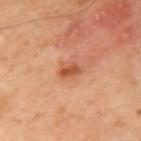biopsy_status: not biopsied; imaged during a skin examination
automated_metrics:
  area_mm2_approx: 3.5
  eccentricity: 0.8
  shape_asymmetry: 0.3
  cielab_L: 52
  cielab_a: 27
  cielab_b: 37
  vs_skin_contrast_norm: 8.0
  lesion_detection_confidence_0_100: 100
lighting: cross-polarized
image:
  source: total-body photography crop
  field_of_view_mm: 15
site: right upper arm
patient:
  sex: male
  age_approx: 65